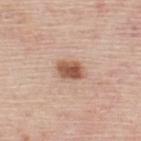Assessment:
Captured during whole-body skin photography for melanoma surveillance; the lesion was not biopsied.
Background:
The lesion is on the back. A male patient, aged 43 to 47. The tile uses white-light illumination. Automated image analysis of the tile measured a lesion area of about 5.5 mm² and two-axis asymmetry of about 0.2. It also reported a mean CIELAB color near L≈55 a*≈22 b*≈30 and about 15 CIELAB-L* units darker than the surrounding skin. It also reported border irregularity of about 2 on a 0–10 scale, internal color variation of about 3.5 on a 0–10 scale, and a peripheral color-asymmetry measure near 1. The software also gave an automated nevus-likeness rating near 95 out of 100 and lesion-presence confidence of about 100/100. The recorded lesion diameter is about 3 mm. A lesion tile, about 15 mm wide, cut from a 3D total-body photograph.